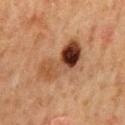follow-up — no biopsy performed (imaged during a skin exam)
acquisition — ~15 mm crop, total-body skin-cancer survey
tile lighting — cross-polarized
location — the mid back
diameter — ≈6.5 mm
subject — male, aged 63 to 67
automated metrics — a lesion area of about 18 mm², an outline eccentricity of about 0.85 (0 = round, 1 = elongated), and two-axis asymmetry of about 0.3; a lesion color around L≈39 a*≈20 b*≈30 in CIELAB